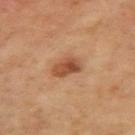Clinical impression: Imaged during a routine full-body skin examination; the lesion was not biopsied and no histopathology is available. Clinical summary: The tile uses cross-polarized illumination. Located on the left arm. A 15 mm close-up extracted from a 3D total-body photography capture. A male subject approximately 65 years of age. Measured at roughly 3.5 mm in maximum diameter.